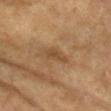biopsy status: no biopsy performed (imaged during a skin exam) | acquisition: total-body-photography crop, ~15 mm field of view | site: the head or neck | patient: female, aged 58–62.